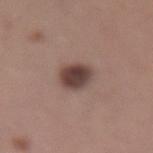Recorded during total-body skin imaging; not selected for excision or biopsy.
A 15 mm close-up tile from a total-body photography series done for melanoma screening.
A female patient aged 28 to 32.
The lesion is located on the right thigh.
Captured under white-light illumination.
The recorded lesion diameter is about 3.5 mm.